Longest diameter approximately 1 mm · the tile uses cross-polarized illumination · a region of skin cropped from a whole-body photographic capture, roughly 15 mm wide · the patient is a male approximately 65 years of age · on the head or neck: 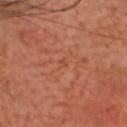{
  "diagnosis": {
    "histopathology": "nodular basal cell carcinoma",
    "malignancy": "malignant",
    "taxonomic_path": [
      "Malignant",
      "Malignant adnexal epithelial proliferations - Follicular",
      "Basal cell carcinoma",
      "Basal cell carcinoma, Nodular"
    ]
  }
}